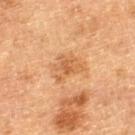Impression: No biopsy was performed on this lesion — it was imaged during a full skin examination and was not determined to be concerning. Clinical summary: A male subject aged around 75. A 15 mm close-up tile from a total-body photography series done for melanoma screening. Approximately 3.5 mm at its widest. This is a cross-polarized tile. Located on the left thigh.